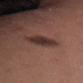The tile uses white-light illumination. Automated tile analysis of the lesion measured a lesion area of about 7.5 mm² and a shape-asymmetry score of about 0.15 (0 = symmetric). The analysis additionally found a mean CIELAB color near L≈33 a*≈19 b*≈20, about 11 CIELAB-L* units darker than the surrounding skin, and a lesion-to-skin contrast of about 10 (normalized; higher = more distinct). It also reported border irregularity of about 2 on a 0–10 scale and a peripheral color-asymmetry measure near 1. The subject is a female approximately 30 years of age. A lesion tile, about 15 mm wide, cut from a 3D total-body photograph. Located on the right thigh.